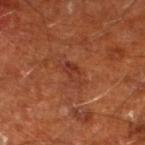Findings:
• notes · total-body-photography surveillance lesion; no biopsy
• tile lighting · cross-polarized illumination
• location · the left lower leg
• automated metrics · a footprint of about 4 mm², an eccentricity of roughly 0.85, and two-axis asymmetry of about 0.45; a lesion color around L≈35 a*≈27 b*≈31 in CIELAB, about 6 CIELAB-L* units darker than the surrounding skin, and a normalized border contrast of about 6; an automated nevus-likeness rating near 0 out of 100
• size · ~3 mm (longest diameter)
• image source · ~15 mm crop, total-body skin-cancer survey
• patient · male, approximately 65 years of age